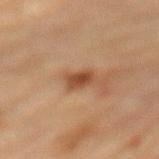follow-up=catalogued during a skin exam; not biopsied | site=the mid back | illumination=cross-polarized | lesion size=about 2.5 mm | TBP lesion metrics=an average lesion color of about L≈40 a*≈20 b*≈30 (CIELAB) and a lesion–skin lightness drop of about 11; peripheral color asymmetry of about 1 | imaging modality=~15 mm crop, total-body skin-cancer survey | subject=female, roughly 80 years of age.